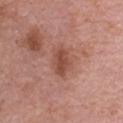| key | value |
|---|---|
| workup | imaged on a skin check; not biopsied |
| automated lesion analysis | an area of roughly 6.5 mm² and a shape-asymmetry score of about 0.2 (0 = symmetric); a border-irregularity rating of about 2/10 and a color-variation rating of about 2/10; a nevus-likeness score of about 15/100 |
| subject | female, aged around 65 |
| tile lighting | white-light illumination |
| image source | total-body-photography crop, ~15 mm field of view |
| anatomic site | the upper back |